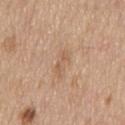| field | value |
|---|---|
| follow-up | no biopsy performed (imaged during a skin exam) |
| lighting | white-light illumination |
| diameter | ≈3 mm |
| subject | male, roughly 70 years of age |
| imaging modality | 15 mm crop, total-body photography |
| anatomic site | the mid back |